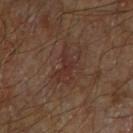<case>
  <biopsy_status>not biopsied; imaged during a skin examination</biopsy_status>
  <site>arm</site>
  <patient>
    <sex>male</sex>
    <age_approx>70</age_approx>
  </patient>
  <image>
    <source>total-body photography crop</source>
    <field_of_view_mm>15</field_of_view_mm>
  </image>
  <automated_metrics>
    <area_mm2_approx>7.5</area_mm2_approx>
    <shape_asymmetry>0.3</shape_asymmetry>
    <cielab_L>30</cielab_L>
    <cielab_a>18</cielab_a>
    <cielab_b>22</cielab_b>
    <border_irregularity_0_10>5.5</border_irregularity_0_10>
    <color_variation_0_10>3.0</color_variation_0_10>
    <peripheral_color_asymmetry>1.0</peripheral_color_asymmetry>
  </automated_metrics>
</case>